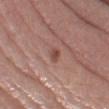notes = no biopsy performed (imaged during a skin exam)
imaging modality = ~15 mm crop, total-body skin-cancer survey
subject = female, approximately 65 years of age
site = the left thigh